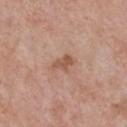{
  "site": "chest",
  "patient": {
    "sex": "female",
    "age_approx": 60
  },
  "lesion_size": {
    "long_diameter_mm_approx": 3.0
  },
  "image": {
    "source": "total-body photography crop",
    "field_of_view_mm": 15
  },
  "lighting": "white-light"
}